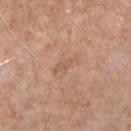Imaged during a routine full-body skin examination; the lesion was not biopsied and no histopathology is available. The subject is a male approximately 65 years of age. This is a white-light tile. A lesion tile, about 15 mm wide, cut from a 3D total-body photograph. On the chest. The lesion's longest dimension is about 3 mm.